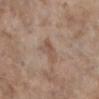Q: Is there a histopathology result?
A: total-body-photography surveillance lesion; no biopsy
Q: What are the patient's age and sex?
A: female, about 85 years old or older
Q: Lesion size?
A: about 3 mm
Q: Automated lesion metrics?
A: a footprint of about 3.5 mm², an eccentricity of roughly 0.85, and a shape-asymmetry score of about 0.3 (0 = symmetric)
Q: What is the anatomic site?
A: the right lower leg
Q: What kind of image is this?
A: ~15 mm crop, total-body skin-cancer survey
Q: What lighting was used for the tile?
A: white-light illumination